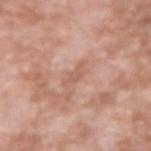Clinical impression: Captured during whole-body skin photography for melanoma surveillance; the lesion was not biopsied. Acquisition and patient details: Measured at roughly 2.5 mm in maximum diameter. The subject is a male aged 58–62. Imaged with white-light lighting. On the right upper arm. An algorithmic analysis of the crop reported a footprint of about 2 mm², an outline eccentricity of about 0.85 (0 = round, 1 = elongated), and a symmetry-axis asymmetry near 0.4. The analysis additionally found a mean CIELAB color near L≈59 a*≈22 b*≈29 and about 7 CIELAB-L* units darker than the surrounding skin. The software also gave a border-irregularity index near 4.5/10. The software also gave an automated nevus-likeness rating near 0 out of 100 and lesion-presence confidence of about 100/100. A 15 mm close-up extracted from a 3D total-body photography capture.